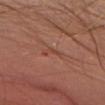Recorded during total-body skin imaging; not selected for excision or biopsy. A female subject approximately 60 years of age. Automated tile analysis of the lesion measured border irregularity of about 6.5 on a 0–10 scale, internal color variation of about 0 on a 0–10 scale, and a peripheral color-asymmetry measure near 0. The lesion is located on the head or neck. A close-up tile cropped from a whole-body skin photograph, about 15 mm across. The tile uses white-light illumination. Measured at roughly 2.5 mm in maximum diameter.